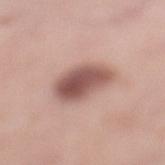| feature | finding |
|---|---|
| automated metrics | an area of roughly 14 mm², an eccentricity of roughly 0.6, and a symmetry-axis asymmetry near 0.15; border irregularity of about 1.5 on a 0–10 scale, a color-variation rating of about 5.5/10, and a peripheral color-asymmetry measure near 2; a classifier nevus-likeness of about 65/100 and a lesion-detection confidence of about 100/100 |
| location | the left lower leg |
| subject | female, in their 50s |
| lesion size | about 5 mm |
| acquisition | total-body-photography crop, ~15 mm field of view |